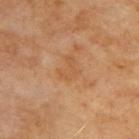Notes:
– follow-up: catalogued during a skin exam; not biopsied
– site: the upper back
– patient: male, about 70 years old
– automated metrics: a footprint of about 4 mm² and an eccentricity of roughly 0.7
– imaging modality: total-body-photography crop, ~15 mm field of view